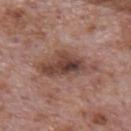<tbp_lesion>
  <biopsy_status>not biopsied; imaged during a skin examination</biopsy_status>
  <patient>
    <sex>male</sex>
    <age_approx>70</age_approx>
  </patient>
  <site>mid back</site>
  <lighting>white-light</lighting>
  <image>
    <source>total-body photography crop</source>
    <field_of_view_mm>15</field_of_view_mm>
  </image>
  <automated_metrics>
    <lesion_detection_confidence_0_100>100</lesion_detection_confidence_0_100>
  </automated_metrics>
</tbp_lesion>